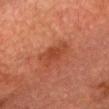This lesion was catalogued during total-body skin photography and was not selected for biopsy.
A roughly 15 mm field-of-view crop from a total-body skin photograph.
The lesion is on the chest.
Imaged with cross-polarized lighting.
A male patient, aged approximately 75.